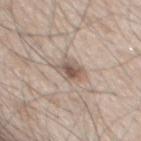• follow-up — imaged on a skin check; not biopsied
• imaging modality — 15 mm crop, total-body photography
• location — the chest
• lesion size — about 3 mm
• image-analysis metrics — an area of roughly 6.5 mm², a shape eccentricity near 0.4, and a shape-asymmetry score of about 0.2 (0 = symmetric); a mean CIELAB color near L≈57 a*≈14 b*≈24, about 11 CIELAB-L* units darker than the surrounding skin, and a lesion-to-skin contrast of about 7.5 (normalized; higher = more distinct); a border-irregularity rating of about 2/10 and a peripheral color-asymmetry measure near 1.5
• patient — male, aged 63 to 67